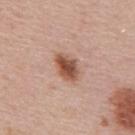Approximately 3.5 mm at its widest.
This image is a 15 mm lesion crop taken from a total-body photograph.
The subject is a male aged 53–57.
Located on the upper back.